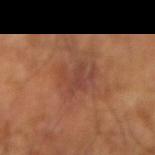Clinical impression: Captured during whole-body skin photography for melanoma surveillance; the lesion was not biopsied. Background: Captured under cross-polarized illumination. A male subject approximately 65 years of age. Approximately 4 mm at its widest. The lesion-visualizer software estimated a border-irregularity index near 5.5/10 and radial color variation of about 1. The software also gave a classifier nevus-likeness of about 0/100. A region of skin cropped from a whole-body photographic capture, roughly 15 mm wide.